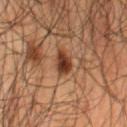workup — imaged on a skin check; not biopsied
automated metrics — a lesion area of about 4.5 mm², a shape eccentricity near 0.7, and a shape-asymmetry score of about 0.15 (0 = symmetric)
diameter — ≈2.5 mm
image — total-body-photography crop, ~15 mm field of view
site — the lower back
lighting — cross-polarized
subject — male, aged 48–52